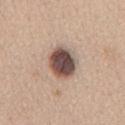Clinical impression:
Part of a total-body skin-imaging series; this lesion was reviewed on a skin check and was not flagged for biopsy.
Image and clinical context:
Captured under white-light illumination. This image is a 15 mm lesion crop taken from a total-body photograph. The lesion is on the mid back. Measured at roughly 4.5 mm in maximum diameter. A female patient aged approximately 40.